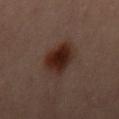Part of a total-body skin-imaging series; this lesion was reviewed on a skin check and was not flagged for biopsy.
Automated tile analysis of the lesion measured a lesion area of about 11 mm², an outline eccentricity of about 0.75 (0 = round, 1 = elongated), and a symmetry-axis asymmetry near 0.2. The analysis additionally found a mean CIELAB color near L≈19 a*≈15 b*≈18 and a normalized lesion–skin contrast near 12.5.
The subject is a male approximately 40 years of age.
On the mid back.
The tile uses cross-polarized illumination.
Cropped from a total-body skin-imaging series; the visible field is about 15 mm.